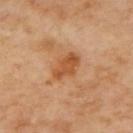follow-up = total-body-photography surveillance lesion; no biopsy
lesion diameter = ~4 mm (longest diameter)
automated metrics = a footprint of about 8 mm² and an eccentricity of roughly 0.75; an average lesion color of about L≈55 a*≈26 b*≈41 (CIELAB), about 10 CIELAB-L* units darker than the surrounding skin, and a normalized lesion–skin contrast near 7.5
imaging modality = ~15 mm tile from a whole-body skin photo
body site = the back